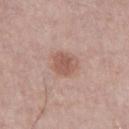biopsy status=no biopsy performed (imaged during a skin exam) | image source=15 mm crop, total-body photography | patient=male, roughly 75 years of age | lighting=white-light illumination | size=about 3 mm | location=the right thigh | TBP lesion metrics=border irregularity of about 2 on a 0–10 scale, a within-lesion color-variation index near 2.5/10, and radial color variation of about 1.